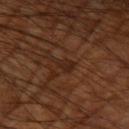– workup · catalogued during a skin exam; not biopsied
– body site · the arm
– image · total-body-photography crop, ~15 mm field of view
– illumination · cross-polarized
– subject · male, about 60 years old
– diameter · ~2.5 mm (longest diameter)